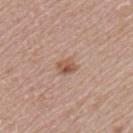Q: Was a biopsy performed?
A: total-body-photography surveillance lesion; no biopsy
Q: Lesion size?
A: ≈2.5 mm
Q: Lesion location?
A: the left upper arm
Q: Illumination type?
A: white-light illumination
Q: How was this image acquired?
A: total-body-photography crop, ~15 mm field of view
Q: Who is the patient?
A: female, approximately 60 years of age
Q: Automated lesion metrics?
A: an area of roughly 4 mm², an outline eccentricity of about 0.65 (0 = round, 1 = elongated), and two-axis asymmetry of about 0.3; an average lesion color of about L≈55 a*≈19 b*≈29 (CIELAB) and about 10 CIELAB-L* units darker than the surrounding skin; a detector confidence of about 100 out of 100 that the crop contains a lesion